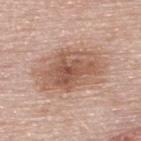Q: Is there a histopathology result?
A: no biopsy performed (imaged during a skin exam)
Q: How was this image acquired?
A: ~15 mm tile from a whole-body skin photo
Q: What are the patient's age and sex?
A: female, roughly 50 years of age
Q: Lesion location?
A: the upper back
Q: Lesion size?
A: about 8 mm